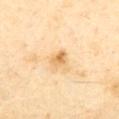Q: Is there a histopathology result?
A: no biopsy performed (imaged during a skin exam)
Q: How was this image acquired?
A: 15 mm crop, total-body photography
Q: What are the patient's age and sex?
A: male, about 70 years old
Q: Lesion size?
A: about 2.5 mm
Q: What is the anatomic site?
A: the abdomen
Q: What lighting was used for the tile?
A: cross-polarized illumination
Q: Automated lesion metrics?
A: a footprint of about 4 mm²